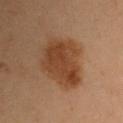follow-up = no biopsy performed (imaged during a skin exam) | tile lighting = cross-polarized | image source = ~15 mm crop, total-body skin-cancer survey | patient = female, in their 40s | body site = the left upper arm.